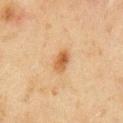Clinical impression: Recorded during total-body skin imaging; not selected for excision or biopsy. Acquisition and patient details: The lesion is located on the left upper arm. A region of skin cropped from a whole-body photographic capture, roughly 15 mm wide. A male subject roughly 45 years of age.